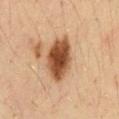This lesion was catalogued during total-body skin photography and was not selected for biopsy. A male patient aged approximately 35. This is a cross-polarized tile. From the chest. Cropped from a total-body skin-imaging series; the visible field is about 15 mm. An algorithmic analysis of the crop reported an area of roughly 14 mm² and two-axis asymmetry of about 0.15.